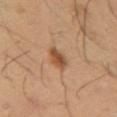{
  "biopsy_status": "not biopsied; imaged during a skin examination",
  "image": {
    "source": "total-body photography crop",
    "field_of_view_mm": 15
  },
  "site": "left forearm",
  "lighting": "cross-polarized",
  "patient": {
    "sex": "male",
    "age_approx": 40
  },
  "lesion_size": {
    "long_diameter_mm_approx": 3.5
  }
}